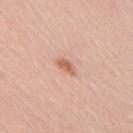- workup — no biopsy performed (imaged during a skin exam)
- body site — the right upper arm
- image source — total-body-photography crop, ~15 mm field of view
- subject — male, roughly 30 years of age
- lighting — white-light